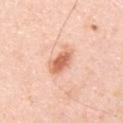Imaged during a routine full-body skin examination; the lesion was not biopsied and no histopathology is available.
Imaged with white-light lighting.
A male patient roughly 35 years of age.
Located on the back.
An algorithmic analysis of the crop reported a within-lesion color-variation index near 4.5/10 and a peripheral color-asymmetry measure near 1. And it measured lesion-presence confidence of about 100/100.
The recorded lesion diameter is about 3.5 mm.
Cropped from a whole-body photographic skin survey; the tile spans about 15 mm.